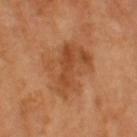Q: Was this lesion biopsied?
A: catalogued during a skin exam; not biopsied
Q: What are the patient's age and sex?
A: female, in their mid- to late 50s
Q: Lesion location?
A: the leg
Q: What is the lesion's diameter?
A: ≈7 mm
Q: How was this image acquired?
A: total-body-photography crop, ~15 mm field of view
Q: What did automated image analysis measure?
A: a mean CIELAB color near L≈50 a*≈25 b*≈39 and about 9 CIELAB-L* units darker than the surrounding skin; a lesion-detection confidence of about 100/100
Q: Illumination type?
A: cross-polarized